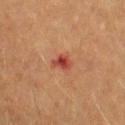No biopsy was performed on this lesion — it was imaged during a full skin examination and was not determined to be concerning.